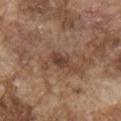This lesion was catalogued during total-body skin photography and was not selected for biopsy.